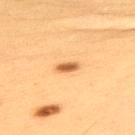biopsy_status: not biopsied; imaged during a skin examination